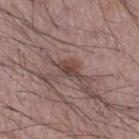The lesion was photographed on a routine skin check and not biopsied; there is no pathology result. Located on the right thigh. A 15 mm crop from a total-body photograph taken for skin-cancer surveillance. A male patient, about 50 years old.